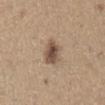The lesion was photographed on a routine skin check and not biopsied; there is no pathology result.
Located on the abdomen.
This image is a 15 mm lesion crop taken from a total-body photograph.
The subject is a male aged around 65.